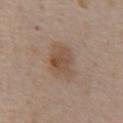Impression: The lesion was tiled from a total-body skin photograph and was not biopsied. Clinical summary: Captured under white-light illumination. Longest diameter approximately 3.5 mm. A region of skin cropped from a whole-body photographic capture, roughly 15 mm wide. The subject is a male aged 58 to 62. Located on the abdomen.